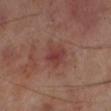workup: imaged on a skin check; not biopsied | diameter: ≈3 mm | site: the left lower leg | illumination: cross-polarized | image source: 15 mm crop, total-body photography | subject: male, approximately 70 years of age | image-analysis metrics: an area of roughly 6 mm² and a shape-asymmetry score of about 0.2 (0 = symmetric); a mean CIELAB color near L≈38 a*≈25 b*≈22, roughly 7 lightness units darker than nearby skin, and a lesion-to-skin contrast of about 6.5 (normalized; higher = more distinct).